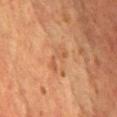Recorded during total-body skin imaging; not selected for excision or biopsy.
The subject is a male aged 58 to 62.
Automated tile analysis of the lesion measured a lesion area of about 4.5 mm² and an eccentricity of roughly 0.9. It also reported roughly 6 lightness units darker than nearby skin. It also reported a nevus-likeness score of about 0/100 and lesion-presence confidence of about 100/100.
The recorded lesion diameter is about 3.5 mm.
The tile uses cross-polarized illumination.
A 15 mm close-up extracted from a 3D total-body photography capture.